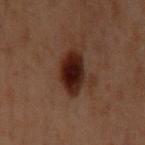| field | value |
|---|---|
| follow-up | no biopsy performed (imaged during a skin exam) |
| image source | ~15 mm tile from a whole-body skin photo |
| site | the left upper arm |
| patient | male, in their 60s |
| automated lesion analysis | a lesion–skin lightness drop of about 11 and a normalized lesion–skin contrast near 14 |
| diameter | ~4 mm (longest diameter) |